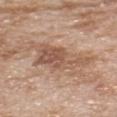Recorded during total-body skin imaging; not selected for excision or biopsy.
Cropped from a total-body skin-imaging series; the visible field is about 15 mm.
A male subject, approximately 80 years of age.
The lesion-visualizer software estimated a footprint of about 16 mm², an outline eccentricity of about 0.85 (0 = round, 1 = elongated), and a symmetry-axis asymmetry near 0.4. It also reported about 10 CIELAB-L* units darker than the surrounding skin and a lesion-to-skin contrast of about 6.5 (normalized; higher = more distinct). It also reported a border-irregularity rating of about 6/10, a color-variation rating of about 5/10, and a peripheral color-asymmetry measure near 1.5. The analysis additionally found a detector confidence of about 100 out of 100 that the crop contains a lesion.
On the upper back.
Measured at roughly 7.5 mm in maximum diameter.